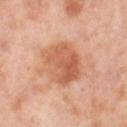<tbp_lesion>
  <biopsy_status>not biopsied; imaged during a skin examination</biopsy_status>
  <patient>
    <sex>female</sex>
    <age_approx>55</age_approx>
  </patient>
  <image>
    <source>total-body photography crop</source>
    <field_of_view_mm>15</field_of_view_mm>
  </image>
  <site>right lower leg</site>
</tbp_lesion>